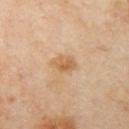Notes:
* biopsy status: no biopsy performed (imaged during a skin exam)
* location: the left upper arm
* image: 15 mm crop, total-body photography
* illumination: cross-polarized
* patient: female, roughly 40 years of age
* lesion size: ~3 mm (longest diameter)
* automated lesion analysis: a lesion color around L≈62 a*≈19 b*≈39 in CIELAB, about 9 CIELAB-L* units darker than the surrounding skin, and a normalized lesion–skin contrast near 7; a border-irregularity rating of about 2/10 and a color-variation rating of about 3/10; a nevus-likeness score of about 5/100 and lesion-presence confidence of about 100/100